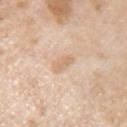| field | value |
|---|---|
| biopsy status | imaged on a skin check; not biopsied |
| image | total-body-photography crop, ~15 mm field of view |
| automated metrics | an average lesion color of about L≈70 a*≈17 b*≈33 (CIELAB) and about 8 CIELAB-L* units darker than the surrounding skin; a peripheral color-asymmetry measure near 0.5; a nevus-likeness score of about 0/100 and a detector confidence of about 100 out of 100 that the crop contains a lesion |
| diameter | about 3 mm |
| site | the left upper arm |
| subject | male, aged around 60 |